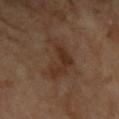| key | value |
|---|---|
| workup | no biopsy performed (imaged during a skin exam) |
| patient | female, aged 68 to 72 |
| location | the left forearm |
| automated lesion analysis | a lesion color around L≈33 a*≈18 b*≈27 in CIELAB, about 7 CIELAB-L* units darker than the surrounding skin, and a normalized lesion–skin contrast near 7; a nevus-likeness score of about 15/100 and a lesion-detection confidence of about 100/100 |
| image source | ~15 mm tile from a whole-body skin photo |
| diameter | ≈5.5 mm |